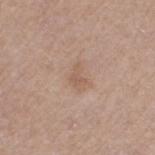{
  "biopsy_status": "not biopsied; imaged during a skin examination",
  "image": {
    "source": "total-body photography crop",
    "field_of_view_mm": 15
  },
  "site": "left thigh",
  "lighting": "white-light",
  "automated_metrics": {
    "area_mm2_approx": 3.0,
    "eccentricity": 0.85,
    "shape_asymmetry": 0.5,
    "cielab_L": 57,
    "cielab_a": 18,
    "cielab_b": 28,
    "vs_skin_contrast_norm": 5.0,
    "border_irregularity_0_10": 5.0,
    "color_variation_0_10": 0.5,
    "peripheral_color_asymmetry": 0.5,
    "nevus_likeness_0_100": 0,
    "lesion_detection_confidence_0_100": 100
  },
  "lesion_size": {
    "long_diameter_mm_approx": 2.5
  },
  "patient": {
    "sex": "female",
    "age_approx": 55
  }
}